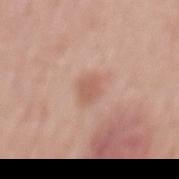Findings:
• notes: total-body-photography surveillance lesion; no biopsy
• subject: male, approximately 70 years of age
• image: ~15 mm tile from a whole-body skin photo
• anatomic site: the mid back